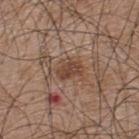TBP lesion metrics = an area of roughly 6 mm² and a shape eccentricity near 0.7; a lesion color around L≈44 a*≈19 b*≈28 in CIELAB, roughly 9 lightness units darker than nearby skin, and a normalized border contrast of about 7; a border-irregularity rating of about 3/10, a color-variation rating of about 2.5/10, and a peripheral color-asymmetry measure near 1; a lesion-detection confidence of about 85/100
acquisition = total-body-photography crop, ~15 mm field of view
lighting = white-light
patient = male, approximately 45 years of age
site = the upper back